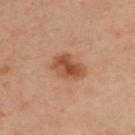Assessment: Recorded during total-body skin imaging; not selected for excision or biopsy. Image and clinical context: The tile uses cross-polarized illumination. A male subject, aged around 45. On the upper back. Automated tile analysis of the lesion measured a lesion area of about 8.5 mm², a shape eccentricity near 0.75, and a symmetry-axis asymmetry near 0.2. The analysis additionally found a lesion color around L≈50 a*≈24 b*≈34 in CIELAB and a lesion–skin lightness drop of about 11. And it measured a border-irregularity index near 2.5/10 and radial color variation of about 1.5. It also reported an automated nevus-likeness rating near 90 out of 100. Longest diameter approximately 4 mm. Cropped from a total-body skin-imaging series; the visible field is about 15 mm.